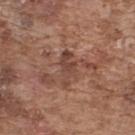Imaged during a routine full-body skin examination; the lesion was not biopsied and no histopathology is available. Located on the upper back. This image is a 15 mm lesion crop taken from a total-body photograph. A male patient, aged approximately 75.